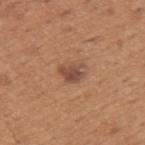Imaged during a routine full-body skin examination; the lesion was not biopsied and no histopathology is available. Approximately 3 mm at its widest. An algorithmic analysis of the crop reported an area of roughly 5.5 mm², a shape eccentricity near 0.65, and a shape-asymmetry score of about 0.35 (0 = symmetric). The analysis additionally found border irregularity of about 4 on a 0–10 scale, a color-variation rating of about 3/10, and a peripheral color-asymmetry measure near 1. A male patient roughly 65 years of age. A 15 mm close-up tile from a total-body photography series done for melanoma screening. The lesion is on the back.